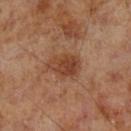Part of a total-body skin-imaging series; this lesion was reviewed on a skin check and was not flagged for biopsy. Imaged with cross-polarized lighting. The lesion is on the left lower leg. A male patient, approximately 70 years of age. A region of skin cropped from a whole-body photographic capture, roughly 15 mm wide. Approximately 4 mm at its widest.